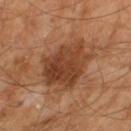biopsy_status: not biopsied; imaged during a skin examination
image:
  source: total-body photography crop
  field_of_view_mm: 15
patient:
  sex: male
  age_approx: 65
lighting: cross-polarized
lesion_size:
  long_diameter_mm_approx: 7.0